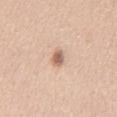Part of a total-body skin-imaging series; this lesion was reviewed on a skin check and was not flagged for biopsy. Automated tile analysis of the lesion measured a border-irregularity rating of about 2/10, a color-variation rating of about 3/10, and peripheral color asymmetry of about 1. The software also gave a lesion-detection confidence of about 100/100. A male patient aged 43–47. The tile uses white-light illumination. A roughly 15 mm field-of-view crop from a total-body skin photograph. Located on the abdomen.